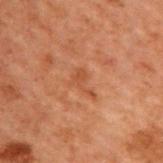{
  "biopsy_status": "not biopsied; imaged during a skin examination",
  "site": "upper back",
  "lesion_size": {
    "long_diameter_mm_approx": 3.0
  },
  "patient": {
    "sex": "male",
    "age_approx": 70
  },
  "image": {
    "source": "total-body photography crop",
    "field_of_view_mm": 15
  },
  "lighting": "cross-polarized"
}